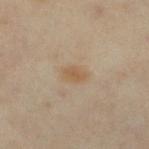Part of a total-body skin-imaging series; this lesion was reviewed on a skin check and was not flagged for biopsy. Automated image analysis of the tile measured a footprint of about 4 mm², an eccentricity of roughly 0.75, and a shape-asymmetry score of about 0.2 (0 = symmetric). The analysis additionally found an average lesion color of about L≈53 a*≈14 b*≈31 (CIELAB) and about 7 CIELAB-L* units darker than the surrounding skin. And it measured border irregularity of about 2 on a 0–10 scale, a within-lesion color-variation index near 2/10, and a peripheral color-asymmetry measure near 0.5. The software also gave an automated nevus-likeness rating near 50 out of 100 and lesion-presence confidence of about 100/100. On the right lower leg. Cropped from a whole-body photographic skin survey; the tile spans about 15 mm. A female patient, approximately 35 years of age. The tile uses cross-polarized illumination.